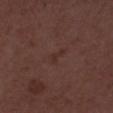biopsy status: imaged on a skin check; not biopsied
image source: ~15 mm tile from a whole-body skin photo
subject: male, aged approximately 50